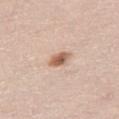{
  "biopsy_status": "not biopsied; imaged during a skin examination",
  "image": {
    "source": "total-body photography crop",
    "field_of_view_mm": 15
  },
  "lesion_size": {
    "long_diameter_mm_approx": 3.0
  },
  "site": "right thigh",
  "patient": {
    "sex": "female",
    "age_approx": 30
  },
  "lighting": "white-light",
  "automated_metrics": {
    "area_mm2_approx": 4.0,
    "shape_asymmetry": 0.3,
    "border_irregularity_0_10": 2.5,
    "color_variation_0_10": 5.5,
    "peripheral_color_asymmetry": 2.0,
    "lesion_detection_confidence_0_100": 100
  }
}